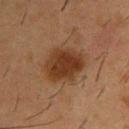<case>
  <biopsy_status>not biopsied; imaged during a skin examination</biopsy_status>
</case>